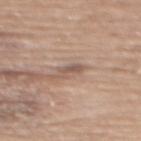Recorded during total-body skin imaging; not selected for excision or biopsy. A female patient, roughly 70 years of age. The lesion is on the upper back. A lesion tile, about 15 mm wide, cut from a 3D total-body photograph.